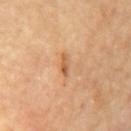Acquisition and patient details: A male subject, aged 68 to 72. The recorded lesion diameter is about 2.5 mm. A region of skin cropped from a whole-body photographic capture, roughly 15 mm wide. Imaged with cross-polarized lighting. Located on the mid back.